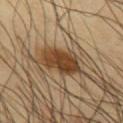{
  "biopsy_status": "not biopsied; imaged during a skin examination",
  "patient": {
    "sex": "male",
    "age_approx": 40
  },
  "lesion_size": {
    "long_diameter_mm_approx": 5.5
  },
  "lighting": "cross-polarized",
  "image": {
    "source": "total-body photography crop",
    "field_of_view_mm": 15
  },
  "site": "chest"
}